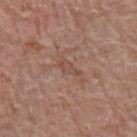biopsy status = catalogued during a skin exam; not biopsied